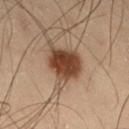<tbp_lesion>
  <biopsy_status>not biopsied; imaged during a skin examination</biopsy_status>
  <site>right thigh</site>
  <image>
    <source>total-body photography crop</source>
    <field_of_view_mm>15</field_of_view_mm>
  </image>
  <lighting>cross-polarized</lighting>
  <patient>
    <sex>male</sex>
    <age_approx>55</age_approx>
  </patient>
</tbp_lesion>